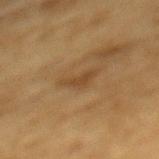Assessment:
No biopsy was performed on this lesion — it was imaged during a full skin examination and was not determined to be concerning.
Clinical summary:
An algorithmic analysis of the crop reported a lesion color around L≈38 a*≈15 b*≈32 in CIELAB and a normalized lesion–skin contrast near 6. It also reported a border-irregularity index near 5/10 and a color-variation rating of about 0.5/10. This is a cross-polarized tile. Located on the mid back. Cropped from a whole-body photographic skin survey; the tile spans about 15 mm. About 3 mm across. The subject is a male aged 83 or older.